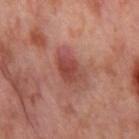Findings:
– notes · no biopsy performed (imaged during a skin exam)
– image-analysis metrics · a footprint of about 8.5 mm², a shape eccentricity near 0.75, and a shape-asymmetry score of about 0.2 (0 = symmetric)
– location · the right thigh
– image source · 15 mm crop, total-body photography
– patient · female, aged around 55
– lighting · cross-polarized illumination